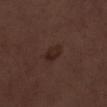Impression:
Recorded during total-body skin imaging; not selected for excision or biopsy.
Clinical summary:
A male patient, in their 70s. On the left lower leg. Imaged with white-light lighting. Automated tile analysis of the lesion measured a lesion color around L≈24 a*≈16 b*≈20 in CIELAB, roughly 6 lightness units darker than nearby skin, and a normalized lesion–skin contrast near 7. The analysis additionally found a color-variation rating of about 3/10 and a peripheral color-asymmetry measure near 1. The analysis additionally found a classifier nevus-likeness of about 65/100 and lesion-presence confidence of about 100/100. Approximately 3 mm at its widest. A 15 mm crop from a total-body photograph taken for skin-cancer surveillance.